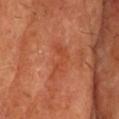{
  "biopsy_status": "not biopsied; imaged during a skin examination",
  "automated_metrics": {
    "cielab_L": 48,
    "cielab_a": 32,
    "cielab_b": 38,
    "vs_skin_darker_L": 6.0,
    "vs_skin_contrast_norm": 5.0
  },
  "image": {
    "source": "total-body photography crop",
    "field_of_view_mm": 15
  },
  "lighting": "cross-polarized",
  "lesion_size": {
    "long_diameter_mm_approx": 4.5
  },
  "patient": {
    "sex": "male",
    "age_approx": 65
  },
  "site": "head or neck"
}